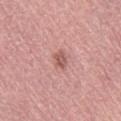Imaged during a routine full-body skin examination; the lesion was not biopsied and no histopathology is available.